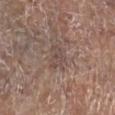Part of a total-body skin-imaging series; this lesion was reviewed on a skin check and was not flagged for biopsy. A male subject, roughly 70 years of age. Measured at roughly 3 mm in maximum diameter. Captured under white-light illumination. The lesion is on the right lower leg. A region of skin cropped from a whole-body photographic capture, roughly 15 mm wide. An algorithmic analysis of the crop reported an outline eccentricity of about 0.7 (0 = round, 1 = elongated) and a shape-asymmetry score of about 0.45 (0 = symmetric). The software also gave an average lesion color of about L≈47 a*≈15 b*≈21 (CIELAB), a lesion–skin lightness drop of about 6, and a normalized lesion–skin contrast near 5. The analysis additionally found a nevus-likeness score of about 0/100 and a lesion-detection confidence of about 80/100.